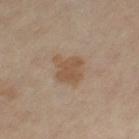Impression: The lesion was photographed on a routine skin check and not biopsied; there is no pathology result. Background: This is a cross-polarized tile. A female subject approximately 50 years of age. Cropped from a whole-body photographic skin survey; the tile spans about 15 mm. Located on the left thigh. About 4 mm across.